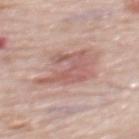notes: total-body-photography surveillance lesion; no biopsy | lesion size: about 5 mm | imaging modality: ~15 mm tile from a whole-body skin photo | illumination: white-light illumination | patient: female, about 65 years old | anatomic site: the upper back.